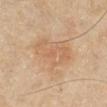Captured during whole-body skin photography for melanoma surveillance; the lesion was not biopsied.
A 15 mm crop from a total-body photograph taken for skin-cancer surveillance.
An algorithmic analysis of the crop reported an area of roughly 19 mm², an outline eccentricity of about 0.75 (0 = round, 1 = elongated), and a shape-asymmetry score of about 0.4 (0 = symmetric). The analysis additionally found an automated nevus-likeness rating near 0 out of 100 and a lesion-detection confidence of about 100/100.
Located on the right lower leg.
A female subject approximately 70 years of age.